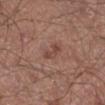Clinical impression: Part of a total-body skin-imaging series; this lesion was reviewed on a skin check and was not flagged for biopsy. Image and clinical context: The recorded lesion diameter is about 2.5 mm. The lesion is located on the leg. The patient is a male roughly 50 years of age. The total-body-photography lesion software estimated an average lesion color of about L≈45 a*≈21 b*≈25 (CIELAB), roughly 8 lightness units darker than nearby skin, and a lesion-to-skin contrast of about 6.5 (normalized; higher = more distinct). The analysis additionally found a nevus-likeness score of about 0/100 and a detector confidence of about 100 out of 100 that the crop contains a lesion. A 15 mm close-up extracted from a 3D total-body photography capture.